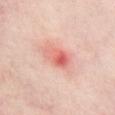Notes:
* size: ~4 mm (longest diameter)
* patient: female, in their mid-50s
* lighting: cross-polarized illumination
* site: the chest
* imaging modality: total-body-photography crop, ~15 mm field of view